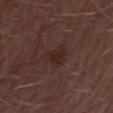The lesion was photographed on a routine skin check and not biopsied; there is no pathology result. Longest diameter approximately 3 mm. The lesion is on the left thigh. Captured under white-light illumination. A close-up tile cropped from a whole-body skin photograph, about 15 mm across. A male subject aged 68 to 72.